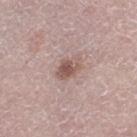<record>
<biopsy_status>not biopsied; imaged during a skin examination</biopsy_status>
<patient>
  <sex>female</sex>
  <age_approx>65</age_approx>
</patient>
<site>leg</site>
<lighting>white-light</lighting>
<image>
  <source>total-body photography crop</source>
  <field_of_view_mm>15</field_of_view_mm>
</image>
<lesion_size>
  <long_diameter_mm_approx>3.0</long_diameter_mm_approx>
</lesion_size>
</record>